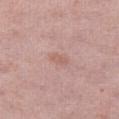This lesion was catalogued during total-body skin photography and was not selected for biopsy.
This image is a 15 mm lesion crop taken from a total-body photograph.
Measured at roughly 2.5 mm in maximum diameter.
A female patient, in their 60s.
Located on the leg.
Captured under white-light illumination.